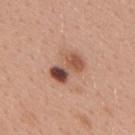Clinical impression:
Captured during whole-body skin photography for melanoma surveillance; the lesion was not biopsied.
Context:
A lesion tile, about 15 mm wide, cut from a 3D total-body photograph. The lesion is located on the back. Longest diameter approximately 4 mm. A female patient, aged approximately 40. This is a white-light tile.